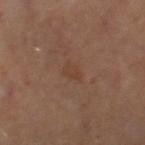Assessment:
Imaged during a routine full-body skin examination; the lesion was not biopsied and no histopathology is available.
Clinical summary:
The total-body-photography lesion software estimated a lesion-detection confidence of about 100/100. Captured under cross-polarized illumination. A lesion tile, about 15 mm wide, cut from a 3D total-body photograph. A female subject, about 50 years old. Located on the right thigh.